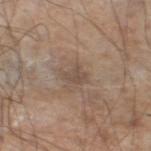Impression:
No biopsy was performed on this lesion — it was imaged during a full skin examination and was not determined to be concerning.
Context:
Located on the left lower leg. A 15 mm close-up extracted from a 3D total-body photography capture. Measured at roughly 2.5 mm in maximum diameter. The tile uses white-light illumination. A male patient aged 58 to 62.